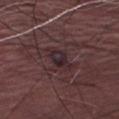Imaged during a routine full-body skin examination; the lesion was not biopsied and no histopathology is available. The patient is a male roughly 75 years of age. The tile uses white-light illumination. The recorded lesion diameter is about 4.5 mm. On the left thigh. An algorithmic analysis of the crop reported a lesion area of about 7.5 mm², an outline eccentricity of about 0.8 (0 = round, 1 = elongated), and two-axis asymmetry of about 0.35. And it measured an average lesion color of about L≈26 a*≈17 b*≈12 (CIELAB), roughly 6 lightness units darker than nearby skin, and a normalized border contrast of about 7.5. It also reported a border-irregularity index near 5/10, a color-variation rating of about 5.5/10, and peripheral color asymmetry of about 1.5. The analysis additionally found a classifier nevus-likeness of about 5/100 and a lesion-detection confidence of about 90/100. A close-up tile cropped from a whole-body skin photograph, about 15 mm across.